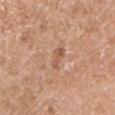The lesion was tiled from a total-body skin photograph and was not biopsied. Cropped from a whole-body photographic skin survey; the tile spans about 15 mm. An algorithmic analysis of the crop reported an area of roughly 4 mm² and a shape-asymmetry score of about 0.25 (0 = symmetric). It also reported about 8 CIELAB-L* units darker than the surrounding skin and a normalized lesion–skin contrast near 5.5. The analysis additionally found a nevus-likeness score of about 0/100 and lesion-presence confidence of about 100/100. Located on the left upper arm. A male patient roughly 55 years of age. The tile uses white-light illumination.